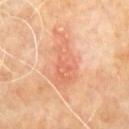The lesion was tiled from a total-body skin photograph and was not biopsied.
A close-up tile cropped from a whole-body skin photograph, about 15 mm across.
A male patient roughly 60 years of age.
Located on the back.